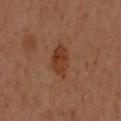This lesion was catalogued during total-body skin photography and was not selected for biopsy.
The subject is a female aged approximately 60.
The lesion is located on the arm.
A region of skin cropped from a whole-body photographic capture, roughly 15 mm wide.